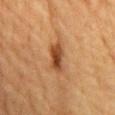Recorded during total-body skin imaging; not selected for excision or biopsy. Located on the front of the torso. The tile uses cross-polarized illumination. A male subject, approximately 85 years of age or older. A 15 mm close-up tile from a total-body photography series done for melanoma screening.